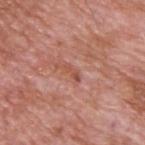{
  "image": {
    "source": "total-body photography crop",
    "field_of_view_mm": 15
  },
  "lighting": "white-light",
  "patient": {
    "sex": "male",
    "age_approx": 60
  },
  "site": "back"
}